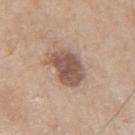Assessment: The lesion was photographed on a routine skin check and not biopsied; there is no pathology result. Acquisition and patient details: Cropped from a total-body skin-imaging series; the visible field is about 15 mm. About 4.5 mm across. The lesion-visualizer software estimated an outline eccentricity of about 0.55 (0 = round, 1 = elongated) and a shape-asymmetry score of about 0.25 (0 = symmetric). And it measured border irregularity of about 3 on a 0–10 scale, a color-variation rating of about 3.5/10, and peripheral color asymmetry of about 1. The software also gave an automated nevus-likeness rating near 30 out of 100 and a lesion-detection confidence of about 100/100. The patient is a male aged around 70. On the right upper arm. Captured under white-light illumination.